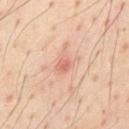The total-body-photography lesion software estimated a border-irregularity index near 3/10, internal color variation of about 3.5 on a 0–10 scale, and radial color variation of about 1. It also reported an automated nevus-likeness rating near 0 out of 100 and lesion-presence confidence of about 100/100.
The recorded lesion diameter is about 3 mm.
The patient is a male in their mid- to late 50s.
The lesion is on the chest.
A 15 mm close-up extracted from a 3D total-body photography capture.